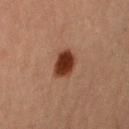Part of a total-body skin-imaging series; this lesion was reviewed on a skin check and was not flagged for biopsy. A female patient aged 58–62. Cropped from a whole-body photographic skin survey; the tile spans about 15 mm. The tile uses cross-polarized illumination. An algorithmic analysis of the crop reported a mean CIELAB color near L≈28 a*≈21 b*≈26, a lesion–skin lightness drop of about 14, and a normalized border contrast of about 13.5. And it measured a within-lesion color-variation index near 3/10. And it measured a detector confidence of about 100 out of 100 that the crop contains a lesion. From the right upper arm. Longest diameter approximately 3.5 mm.